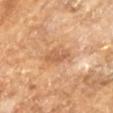notes: imaged on a skin check; not biopsied
size: about 3.5 mm
imaging modality: ~15 mm crop, total-body skin-cancer survey
subject: male, roughly 65 years of age